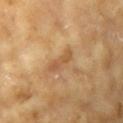The lesion was tiled from a total-body skin photograph and was not biopsied. About 4 mm across. A close-up tile cropped from a whole-body skin photograph, about 15 mm across. The lesion is on the right upper arm. The tile uses cross-polarized illumination. The subject is a female in their mid-70s.